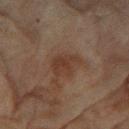Part of a total-body skin-imaging series; this lesion was reviewed on a skin check and was not flagged for biopsy.